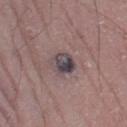Case summary:
* workup · no biopsy performed (imaged during a skin exam)